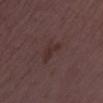Part of a total-body skin-imaging series; this lesion was reviewed on a skin check and was not flagged for biopsy. A 15 mm close-up extracted from a 3D total-body photography capture. Imaged with white-light lighting. The lesion is on the right thigh. A female patient, aged 28 to 32. About 2.5 mm across.